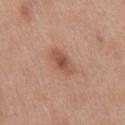Assessment: Recorded during total-body skin imaging; not selected for excision or biopsy. Acquisition and patient details: The subject is a female approximately 40 years of age. Longest diameter approximately 3.5 mm. From the chest. The tile uses white-light illumination. A 15 mm close-up tile from a total-body photography series done for melanoma screening.